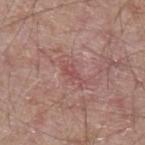notes: total-body-photography surveillance lesion; no biopsy | illumination: white-light illumination | patient: male, in their mid- to late 60s | image source: ~15 mm tile from a whole-body skin photo | size: ~3 mm (longest diameter) | anatomic site: the left forearm.